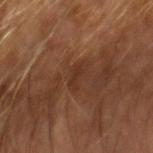Assessment: This lesion was catalogued during total-body skin photography and was not selected for biopsy. Clinical summary: Located on the arm. A male subject, aged 63 to 67. A 15 mm close-up tile from a total-body photography series done for melanoma screening.